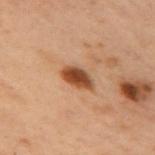Clinical impression: Part of a total-body skin-imaging series; this lesion was reviewed on a skin check and was not flagged for biopsy. Clinical summary: A female subject, in their mid-50s. This image is a 15 mm lesion crop taken from a total-body photograph. Located on the arm.